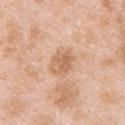A male patient, about 25 years old. A lesion tile, about 15 mm wide, cut from a 3D total-body photograph. The lesion's longest dimension is about 3 mm. The tile uses white-light illumination. On the upper back.